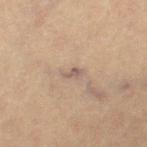Impression: This lesion was catalogued during total-body skin photography and was not selected for biopsy. Background: Imaged with cross-polarized lighting. A male subject, roughly 85 years of age or older. The recorded lesion diameter is about 2.5 mm. Automated image analysis of the tile measured a lesion color around L≈56 a*≈15 b*≈23 in CIELAB, about 8 CIELAB-L* units darker than the surrounding skin, and a normalized border contrast of about 7. A 15 mm close-up tile from a total-body photography series done for melanoma screening. On the right thigh.